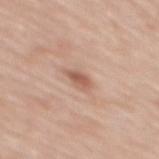follow-up: imaged on a skin check; not biopsied | body site: the upper back | illumination: white-light | patient: female, aged 53–57 | lesion size: ≈2.5 mm | imaging modality: total-body-photography crop, ~15 mm field of view.